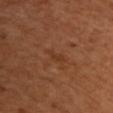Captured during whole-body skin photography for melanoma surveillance; the lesion was not biopsied. The tile uses cross-polarized illumination. The patient is a female aged around 55. A region of skin cropped from a whole-body photographic capture, roughly 15 mm wide. About 2.5 mm across. On the chest.